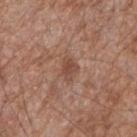Imaged during a routine full-body skin examination; the lesion was not biopsied and no histopathology is available. This image is a 15 mm lesion crop taken from a total-body photograph. From the right forearm. A male patient aged 53–57.